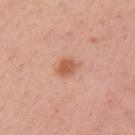{
  "biopsy_status": "not biopsied; imaged during a skin examination",
  "automated_metrics": {
    "cielab_L": 59,
    "cielab_a": 26,
    "cielab_b": 34,
    "vs_skin_darker_L": 10.0,
    "color_variation_0_10": 3.0,
    "peripheral_color_asymmetry": 1.0
  },
  "site": "left upper arm",
  "lighting": "white-light",
  "lesion_size": {
    "long_diameter_mm_approx": 3.0
  },
  "patient": {
    "sex": "female",
    "age_approx": 35
  },
  "image": {
    "source": "total-body photography crop",
    "field_of_view_mm": 15
  }
}